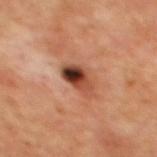Impression: Part of a total-body skin-imaging series; this lesion was reviewed on a skin check and was not flagged for biopsy. Acquisition and patient details: A patient aged approximately 65. Longest diameter approximately 4 mm. A 15 mm close-up tile from a total-body photography series done for melanoma screening. The lesion is on the mid back. Imaged with cross-polarized lighting.